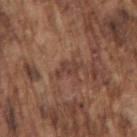Imaged during a routine full-body skin examination; the lesion was not biopsied and no histopathology is available. On the arm. The total-body-photography lesion software estimated an outline eccentricity of about 0.9 (0 = round, 1 = elongated) and two-axis asymmetry of about 0.4. A male patient, about 75 years old. A 15 mm close-up tile from a total-body photography series done for melanoma screening. Captured under white-light illumination.